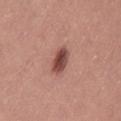workup: catalogued during a skin exam; not biopsied | image-analysis metrics: a border-irregularity rating of about 2.5/10 | size: ~4 mm (longest diameter) | imaging modality: ~15 mm tile from a whole-body skin photo | site: the left thigh | subject: female, in their mid-20s.